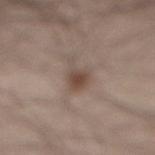| key | value |
|---|---|
| follow-up | total-body-photography surveillance lesion; no biopsy |
| image-analysis metrics | an automated nevus-likeness rating near 70 out of 100 and a detector confidence of about 100 out of 100 that the crop contains a lesion |
| lesion size | about 3.5 mm |
| location | the back |
| acquisition | ~15 mm crop, total-body skin-cancer survey |
| patient | male, in their mid-30s |